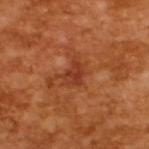Part of a total-body skin-imaging series; this lesion was reviewed on a skin check and was not flagged for biopsy. A region of skin cropped from a whole-body photographic capture, roughly 15 mm wide. This is a cross-polarized tile. The lesion's longest dimension is about 3 mm. A male patient, aged 63–67.